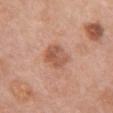notes: imaged on a skin check; not biopsied
patient: female, about 60 years old
acquisition: total-body-photography crop, ~15 mm field of view
body site: the chest